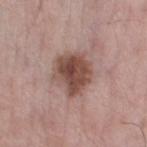Case summary:
* notes · no biopsy performed (imaged during a skin exam)
* lesion diameter · ≈4.5 mm
* patient · male, aged 68 to 72
* illumination · white-light illumination
* image · ~15 mm crop, total-body skin-cancer survey
* image-analysis metrics · an area of roughly 13 mm², a shape eccentricity near 0.55, and a symmetry-axis asymmetry near 0.25; an average lesion color of about L≈47 a*≈20 b*≈24 (CIELAB); a border-irregularity index near 2.5/10 and a color-variation rating of about 5.5/10; a classifier nevus-likeness of about 70/100 and a lesion-detection confidence of about 100/100
* location · the left thigh